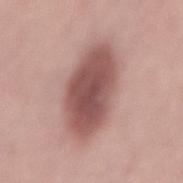* notes — imaged on a skin check; not biopsied
* acquisition — ~15 mm tile from a whole-body skin photo
* automated metrics — a mean CIELAB color near L≈52 a*≈23 b*≈22 and a lesion–skin lightness drop of about 15
* illumination — white-light
* patient — male, aged around 25
* site — the lower back
* lesion diameter — about 8 mm